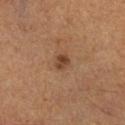Part of a total-body skin-imaging series; this lesion was reviewed on a skin check and was not flagged for biopsy.
Cropped from a whole-body photographic skin survey; the tile spans about 15 mm.
From the leg.
A male subject approximately 65 years of age.
The total-body-photography lesion software estimated an area of roughly 3 mm², an outline eccentricity of about 0.45 (0 = round, 1 = elongated), and a shape-asymmetry score of about 0.3 (0 = symmetric). The analysis additionally found a lesion color around L≈40 a*≈20 b*≈30 in CIELAB. It also reported a classifier nevus-likeness of about 80/100 and a detector confidence of about 100 out of 100 that the crop contains a lesion.
This is a cross-polarized tile.